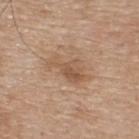Q: Was a biopsy performed?
A: catalogued during a skin exam; not biopsied
Q: How was this image acquired?
A: ~15 mm crop, total-body skin-cancer survey
Q: Where on the body is the lesion?
A: the upper back
Q: Patient demographics?
A: male, aged 73 to 77
Q: Lesion size?
A: ~4.5 mm (longest diameter)